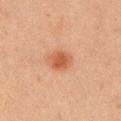notes — total-body-photography surveillance lesion; no biopsy | acquisition — ~15 mm tile from a whole-body skin photo | size — ≈3.5 mm | anatomic site — the left upper arm | patient — female, about 65 years old | illumination — cross-polarized | automated metrics — a classifier nevus-likeness of about 95/100 and a detector confidence of about 100 out of 100 that the crop contains a lesion.